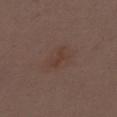Recorded during total-body skin imaging; not selected for excision or biopsy. The tile uses white-light illumination. A female subject, aged 28 to 32. The recorded lesion diameter is about 3 mm. The lesion is on the mid back. A lesion tile, about 15 mm wide, cut from a 3D total-body photograph.